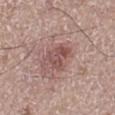Impression:
The lesion was photographed on a routine skin check and not biopsied; there is no pathology result.
Context:
This image is a 15 mm lesion crop taken from a total-body photograph. The lesion is on the left lower leg. Imaged with white-light lighting. A male subject aged 63–67. About 4.5 mm across.